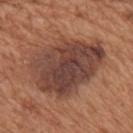Part of a total-body skin-imaging series; this lesion was reviewed on a skin check and was not flagged for biopsy. The subject is a male aged 63–67. The lesion is on the mid back. A 15 mm close-up extracted from a 3D total-body photography capture.